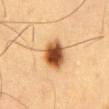Imaged during a routine full-body skin examination; the lesion was not biopsied and no histopathology is available.
The subject is a male roughly 60 years of age.
Longest diameter approximately 4 mm.
A roughly 15 mm field-of-view crop from a total-body skin photograph.
The total-body-photography lesion software estimated a lesion area of about 11 mm², a shape eccentricity near 0.45, and a symmetry-axis asymmetry near 0.15. It also reported border irregularity of about 1.5 on a 0–10 scale, a color-variation rating of about 7.5/10, and a peripheral color-asymmetry measure near 2.
The lesion is located on the abdomen.